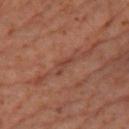Imaged with cross-polarized lighting.
A 15 mm crop from a total-body photograph taken for skin-cancer surveillance.
Longest diameter approximately 2.5 mm.
A male subject, aged 58 to 62.
The lesion is located on the leg.
An algorithmic analysis of the crop reported a lesion area of about 2 mm², an outline eccentricity of about 0.9 (0 = round, 1 = elongated), and two-axis asymmetry of about 0.8. It also reported a lesion-to-skin contrast of about 5.5 (normalized; higher = more distinct).